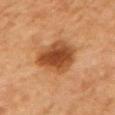Part of a total-body skin-imaging series; this lesion was reviewed on a skin check and was not flagged for biopsy. A 15 mm close-up tile from a total-body photography series done for melanoma screening. Located on the right upper arm. The lesion's longest dimension is about 5 mm. A female patient, aged approximately 55.